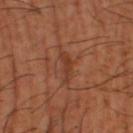Assessment: Imaged during a routine full-body skin examination; the lesion was not biopsied and no histopathology is available. Background: A 15 mm close-up extracted from a 3D total-body photography capture. A male subject, approximately 65 years of age. On the left thigh. The tile uses cross-polarized illumination. Measured at roughly 3.5 mm in maximum diameter.